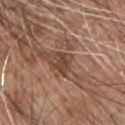{
  "biopsy_status": "not biopsied; imaged during a skin examination",
  "image": {
    "source": "total-body photography crop",
    "field_of_view_mm": 15
  },
  "site": "front of the torso",
  "patient": {
    "sex": "male",
    "age_approx": 45
  },
  "lighting": "white-light",
  "lesion_size": {
    "long_diameter_mm_approx": 2.5
  }
}